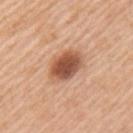Assessment: Captured during whole-body skin photography for melanoma surveillance; the lesion was not biopsied. Context: Located on the left upper arm. Measured at roughly 4 mm in maximum diameter. A lesion tile, about 15 mm wide, cut from a 3D total-body photograph. This is a white-light tile. The patient is a female about 45 years old. The lesion-visualizer software estimated an average lesion color of about L≈54 a*≈24 b*≈33 (CIELAB), about 16 CIELAB-L* units darker than the surrounding skin, and a normalized lesion–skin contrast near 10.